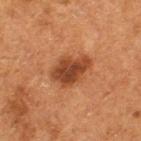| field | value |
|---|---|
| workup | imaged on a skin check; not biopsied |
| acquisition | ~15 mm crop, total-body skin-cancer survey |
| subject | female, aged 48–52 |
| body site | the left thigh |
| automated metrics | an outline eccentricity of about 0.8 (0 = round, 1 = elongated); an average lesion color of about L≈35 a*≈23 b*≈31 (CIELAB), roughly 11 lightness units darker than nearby skin, and a lesion-to-skin contrast of about 10 (normalized; higher = more distinct); a border-irregularity rating of about 2.5/10, internal color variation of about 3.5 on a 0–10 scale, and radial color variation of about 1 |
| lesion diameter | about 4.5 mm |